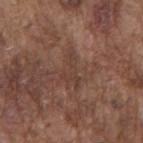{"biopsy_status": "not biopsied; imaged during a skin examination", "patient": {"sex": "male", "age_approx": 75}, "site": "mid back", "lighting": "white-light", "lesion_size": {"long_diameter_mm_approx": 4.0}, "automated_metrics": {"cielab_L": 39, "cielab_a": 18, "cielab_b": 24, "vs_skin_darker_L": 5.0, "nevus_likeness_0_100": 0, "lesion_detection_confidence_0_100": 50}, "image": {"source": "total-body photography crop", "field_of_view_mm": 15}}